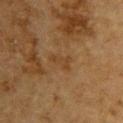Impression: The lesion was photographed on a routine skin check and not biopsied; there is no pathology result. Context: The subject is a male about 85 years old or older. A region of skin cropped from a whole-body photographic capture, roughly 15 mm wide. The lesion is located on the back. Captured under cross-polarized illumination.